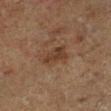A male subject aged around 75. Cropped from a whole-body photographic skin survey; the tile spans about 15 mm. From the right lower leg. The tile uses cross-polarized illumination. About 3.5 mm across. Automated image analysis of the tile measured an area of roughly 6 mm² and an outline eccentricity of about 0.8 (0 = round, 1 = elongated). The analysis additionally found border irregularity of about 4 on a 0–10 scale, a within-lesion color-variation index near 2/10, and a peripheral color-asymmetry measure near 0.5.